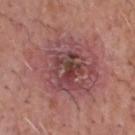Q: Was this lesion biopsied?
A: catalogued during a skin exam; not biopsied
Q: What kind of image is this?
A: 15 mm crop, total-body photography
Q: What is the anatomic site?
A: the head or neck
Q: Who is the patient?
A: male, aged around 60